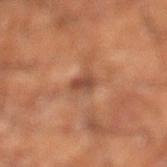Findings:
– workup · no biopsy performed (imaged during a skin exam)
– tile lighting · cross-polarized
– subject · male, approximately 60 years of age
– image source · total-body-photography crop, ~15 mm field of view
– site · the leg
– size · about 2.5 mm